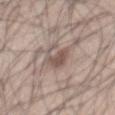workup = total-body-photography surveillance lesion; no biopsy | site = the mid back | imaging modality = ~15 mm crop, total-body skin-cancer survey | size = ~5 mm (longest diameter) | illumination = white-light illumination | subject = male, aged 43–47 | TBP lesion metrics = a lesion color around L≈54 a*≈15 b*≈21 in CIELAB, a lesion–skin lightness drop of about 10, and a normalized lesion–skin contrast near 7.5; a border-irregularity rating of about 5/10 and a within-lesion color-variation index near 2.5/10.